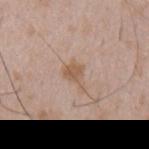Clinical impression:
Captured during whole-body skin photography for melanoma surveillance; the lesion was not biopsied.
Image and clinical context:
Imaged with white-light lighting. About 3 mm across. The patient is a male roughly 50 years of age. An algorithmic analysis of the crop reported a lesion area of about 4.5 mm², an eccentricity of roughly 0.7, and two-axis asymmetry of about 0.45. The analysis additionally found an average lesion color of about L≈57 a*≈17 b*≈29 (CIELAB), about 8 CIELAB-L* units darker than the surrounding skin, and a lesion-to-skin contrast of about 6.5 (normalized; higher = more distinct). And it measured border irregularity of about 5 on a 0–10 scale and a within-lesion color-variation index near 2/10. From the mid back. A close-up tile cropped from a whole-body skin photograph, about 15 mm across.